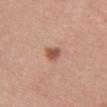Impression: Captured during whole-body skin photography for melanoma surveillance; the lesion was not biopsied. Acquisition and patient details: The lesion is on the upper back. A female patient in their mid- to late 20s. A 15 mm crop from a total-body photograph taken for skin-cancer surveillance. Longest diameter approximately 2.5 mm.